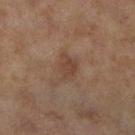Impression:
Imaged during a routine full-body skin examination; the lesion was not biopsied and no histopathology is available.
Context:
A female patient approximately 60 years of age. The lesion-visualizer software estimated a lesion area of about 6 mm² and an outline eccentricity of about 0.7 (0 = round, 1 = elongated). The software also gave a lesion color around L≈36 a*≈16 b*≈23 in CIELAB and a normalized border contrast of about 6. It also reported border irregularity of about 3.5 on a 0–10 scale, a within-lesion color-variation index near 2.5/10, and radial color variation of about 1. On the right lower leg. The lesion's longest dimension is about 3.5 mm. A close-up tile cropped from a whole-body skin photograph, about 15 mm across. The tile uses cross-polarized illumination.